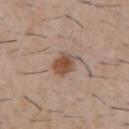Q: Was a biopsy performed?
A: no biopsy performed (imaged during a skin exam)
Q: Patient demographics?
A: male, in their 30s
Q: Where on the body is the lesion?
A: the chest
Q: What is the lesion's diameter?
A: ~3 mm (longest diameter)
Q: Illumination type?
A: white-light
Q: What kind of image is this?
A: ~15 mm crop, total-body skin-cancer survey